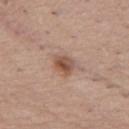Case summary:
– notes · total-body-photography surveillance lesion; no biopsy
– subject · female, approximately 65 years of age
– image source · 15 mm crop, total-body photography
– diameter · about 3 mm
– anatomic site · the front of the torso
– image-analysis metrics · a footprint of about 5 mm² and two-axis asymmetry of about 0.2; a mean CIELAB color near L≈52 a*≈20 b*≈28, roughly 11 lightness units darker than nearby skin, and a normalized border contrast of about 8; a classifier nevus-likeness of about 80/100